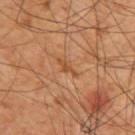Notes:
– workup — imaged on a skin check; not biopsied
– patient — male, approximately 60 years of age
– size — ≈3 mm
– automated metrics — a footprint of about 2.5 mm², an outline eccentricity of about 0.95 (0 = round, 1 = elongated), and a shape-asymmetry score of about 0.45 (0 = symmetric)
– anatomic site — the back
– tile lighting — cross-polarized illumination
– image source — ~15 mm crop, total-body skin-cancer survey